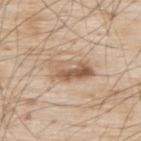The subject is a male approximately 80 years of age. Captured under white-light illumination. Cropped from a whole-body photographic skin survey; the tile spans about 15 mm. The recorded lesion diameter is about 5 mm. Located on the upper back.